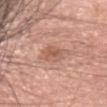  biopsy_status: not biopsied; imaged during a skin examination
  image:
    source: total-body photography crop
    field_of_view_mm: 15
  patient:
    sex: female
    age_approx: 35
  lighting: white-light
  lesion_size:
    long_diameter_mm_approx: 2.5
  site: head or neck
  automated_metrics:
    area_mm2_approx: 4.5
    eccentricity: 0.7
    shape_asymmetry: 0.3